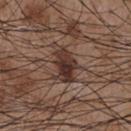This lesion was catalogued during total-body skin photography and was not selected for biopsy. An algorithmic analysis of the crop reported two-axis asymmetry of about 0.3. And it measured a lesion color around L≈32 a*≈17 b*≈22 in CIELAB, about 13 CIELAB-L* units darker than the surrounding skin, and a normalized border contrast of about 11.5. About 4 mm across. The subject is a male in their mid-50s. The lesion is on the chest. A 15 mm crop from a total-body photograph taken for skin-cancer surveillance. The tile uses white-light illumination.